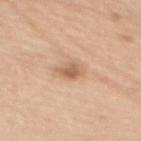<record>
  <biopsy_status>not biopsied; imaged during a skin examination</biopsy_status>
  <image>
    <source>total-body photography crop</source>
    <field_of_view_mm>15</field_of_view_mm>
  </image>
  <automated_metrics>
    <area_mm2_approx>4.5</area_mm2_approx>
    <eccentricity>0.65</eccentricity>
    <shape_asymmetry>0.25</shape_asymmetry>
    <border_irregularity_0_10>2.5</border_irregularity_0_10>
    <color_variation_0_10>3.5</color_variation_0_10>
    <peripheral_color_asymmetry>1.0</peripheral_color_asymmetry>
    <lesion_detection_confidence_0_100>100</lesion_detection_confidence_0_100>
  </automated_metrics>
  <site>mid back</site>
  <lesion_size>
    <long_diameter_mm_approx>3.0</long_diameter_mm_approx>
  </lesion_size>
  <patient>
    <sex>female</sex>
    <age_approx>70</age_approx>
  </patient>
  <lighting>white-light</lighting>
</record>